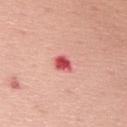Imaged during a routine full-body skin examination; the lesion was not biopsied and no histopathology is available. A lesion tile, about 15 mm wide, cut from a 3D total-body photograph. This is a white-light tile. On the mid back. Approximately 2.5 mm at its widest. The subject is a female aged 63 to 67.